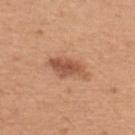biopsy_status: not biopsied; imaged during a skin examination
patient:
  sex: female
  age_approx: 30
lighting: white-light
lesion_size:
  long_diameter_mm_approx: 5.0
site: upper back
image:
  source: total-body photography crop
  field_of_view_mm: 15